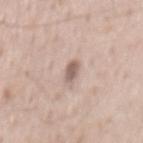• follow-up · no biopsy performed (imaged during a skin exam)
• imaging modality · total-body-photography crop, ~15 mm field of view
• lighting · white-light illumination
• TBP lesion metrics · a nevus-likeness score of about 40/100 and a lesion-detection confidence of about 100/100
• patient · male, about 50 years old
• lesion diameter · about 2.5 mm
• anatomic site · the mid back